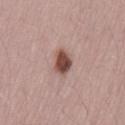workup: catalogued during a skin exam; not biopsied
imaging modality: ~15 mm crop, total-body skin-cancer survey
lesion diameter: ≈3.5 mm
lighting: white-light
subject: male, about 70 years old
body site: the lower back